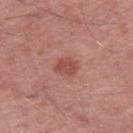{
  "biopsy_status": "not biopsied; imaged during a skin examination",
  "patient": {
    "sex": "male",
    "age_approx": 80
  },
  "image": {
    "source": "total-body photography crop",
    "field_of_view_mm": 15
  },
  "lighting": "white-light",
  "site": "right thigh",
  "automated_metrics": {
    "area_mm2_approx": 4.5,
    "eccentricity": 0.6,
    "shape_asymmetry": 0.2,
    "border_irregularity_0_10": 2.0,
    "color_variation_0_10": 1.5,
    "peripheral_color_asymmetry": 0.5,
    "lesion_detection_confidence_0_100": 100
  }
}